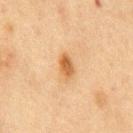{"biopsy_status": "not biopsied; imaged during a skin examination", "patient": {"sex": "male", "age_approx": 75}, "automated_metrics": {"lesion_detection_confidence_0_100": 100}, "image": {"source": "total-body photography crop", "field_of_view_mm": 15}, "site": "chest"}